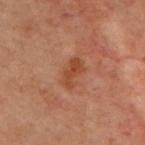{
  "biopsy_status": "not biopsied; imaged during a skin examination"
}